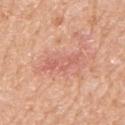No biopsy was performed on this lesion — it was imaged during a full skin examination and was not determined to be concerning. Captured under white-light illumination. On the left upper arm. A 15 mm close-up extracted from a 3D total-body photography capture. A female subject, aged 73 to 77. Automated image analysis of the tile measured an area of roughly 7 mm², an eccentricity of roughly 0.95, and a symmetry-axis asymmetry near 0.45.